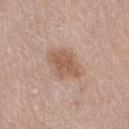Case summary:
– workup — total-body-photography surveillance lesion; no biopsy